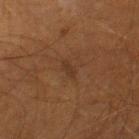No biopsy was performed on this lesion — it was imaged during a full skin examination and was not determined to be concerning.